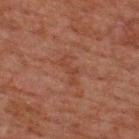  biopsy_status: not biopsied; imaged during a skin examination
  lighting: cross-polarized
  site: upper back
  patient:
    sex: male
    age_approx: 60
  image:
    source: total-body photography crop
    field_of_view_mm: 15